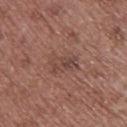The lesion was tiled from a total-body skin photograph and was not biopsied.
The total-body-photography lesion software estimated a lesion area of about 4.5 mm², a shape eccentricity near 0.85, and a shape-asymmetry score of about 0.35 (0 = symmetric). It also reported a border-irregularity index near 5.5/10 and a within-lesion color-variation index near 4/10. The analysis additionally found a nevus-likeness score of about 0/100 and lesion-presence confidence of about 100/100.
This is a white-light tile.
This image is a 15 mm lesion crop taken from a total-body photograph.
On the upper back.
A female subject, aged around 65.